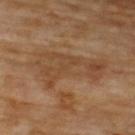Q: How large is the lesion?
A: ≈9 mm
Q: Who is the patient?
A: male, aged 68 to 72
Q: What is the imaging modality?
A: total-body-photography crop, ~15 mm field of view
Q: What did automated image analysis measure?
A: a lesion area of about 23 mm² and a shape-asymmetry score of about 0.45 (0 = symmetric); about 6 CIELAB-L* units darker than the surrounding skin and a lesion-to-skin contrast of about 5 (normalized; higher = more distinct)
Q: Illumination type?
A: cross-polarized
Q: Where on the body is the lesion?
A: the upper back